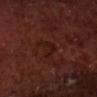Findings:
• notes: total-body-photography surveillance lesion; no biopsy
• body site: the chest
• lesion size: about 3 mm
• acquisition: ~15 mm tile from a whole-body skin photo
• TBP lesion metrics: a mean CIELAB color near L≈17 a*≈20 b*≈21, about 4 CIELAB-L* units darker than the surrounding skin, and a normalized border contrast of about 5.5; border irregularity of about 7.5 on a 0–10 scale, a color-variation rating of about 2.5/10, and a peripheral color-asymmetry measure near 1; a classifier nevus-likeness of about 0/100 and a lesion-detection confidence of about 95/100
• subject: male, aged approximately 70
• lighting: cross-polarized illumination